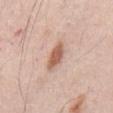Imaged during a routine full-body skin examination; the lesion was not biopsied and no histopathology is available.
The lesion-visualizer software estimated a footprint of about 5.5 mm² and a shape-asymmetry score of about 0.2 (0 = symmetric). And it measured a lesion color around L≈59 a*≈22 b*≈30 in CIELAB, about 14 CIELAB-L* units darker than the surrounding skin, and a normalized lesion–skin contrast near 9. And it measured internal color variation of about 2 on a 0–10 scale.
On the front of the torso.
A 15 mm close-up extracted from a 3D total-body photography capture.
A male patient, aged 53–57.
The tile uses white-light illumination.
The lesion's longest dimension is about 4 mm.